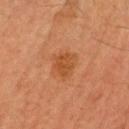Imaged during a routine full-body skin examination; the lesion was not biopsied and no histopathology is available. A close-up tile cropped from a whole-body skin photograph, about 15 mm across. The patient is a male aged 58–62. The total-body-photography lesion software estimated an area of roughly 6 mm². And it measured an average lesion color of about L≈43 a*≈24 b*≈37 (CIELAB), a lesion–skin lightness drop of about 7, and a normalized lesion–skin contrast near 6.5. The analysis additionally found a border-irregularity index near 2.5/10 and radial color variation of about 1. The software also gave a nevus-likeness score of about 35/100 and a lesion-detection confidence of about 100/100. The lesion's longest dimension is about 3 mm.